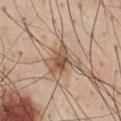{"biopsy_status": "not biopsied; imaged during a skin examination", "patient": {"sex": "male", "age_approx": 60}, "site": "chest", "lesion_size": {"long_diameter_mm_approx": 4.0}, "image": {"source": "total-body photography crop", "field_of_view_mm": 15}, "automated_metrics": {"area_mm2_approx": 5.5, "eccentricity": 0.85, "shape_asymmetry": 0.4, "border_irregularity_0_10": 4.0, "color_variation_0_10": 6.0, "peripheral_color_asymmetry": 2.5}}